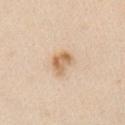No biopsy was performed on this lesion — it was imaged during a full skin examination and was not determined to be concerning.
On the right upper arm.
Captured under white-light illumination.
A male subject aged approximately 60.
Longest diameter approximately 3 mm.
This image is a 15 mm lesion crop taken from a total-body photograph.